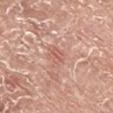Clinical impression:
No biopsy was performed on this lesion — it was imaged during a full skin examination and was not determined to be concerning.
Image and clinical context:
The lesion-visualizer software estimated an outline eccentricity of about 0.8 (0 = round, 1 = elongated). The analysis additionally found peripheral color asymmetry of about 1. The software also gave a nevus-likeness score of about 0/100 and a lesion-detection confidence of about 100/100. Located on the right lower leg. The tile uses white-light illumination. Cropped from a total-body skin-imaging series; the visible field is about 15 mm. A male patient aged 73 to 77.